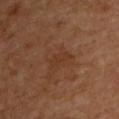Part of a total-body skin-imaging series; this lesion was reviewed on a skin check and was not flagged for biopsy.
Measured at roughly 3 mm in maximum diameter.
A 15 mm crop from a total-body photograph taken for skin-cancer surveillance.
This is a cross-polarized tile.
The lesion is on the upper back.
The subject is a male aged 58 to 62.